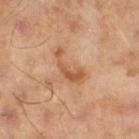| key | value |
|---|---|
| notes | imaged on a skin check; not biopsied |
| acquisition | 15 mm crop, total-body photography |
| size | ≈4.5 mm |
| image-analysis metrics | an area of roughly 6 mm² and two-axis asymmetry of about 0.7 |
| subject | male, approximately 65 years of age |
| illumination | cross-polarized |